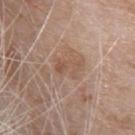  biopsy_status: not biopsied; imaged during a skin examination
  image:
    source: total-body photography crop
    field_of_view_mm: 15
  patient:
    sex: female
    age_approx: 75
  lighting: white-light
  site: upper back
  automated_metrics:
    cielab_L: 53
    cielab_a: 18
    cielab_b: 28
    vs_skin_darker_L: 7.0
    vs_skin_contrast_norm: 5.0
    color_variation_0_10: 1.0
    peripheral_color_asymmetry: 0.5
    nevus_likeness_0_100: 0
    lesion_detection_confidence_0_100: 100
  lesion_size:
    long_diameter_mm_approx: 3.0